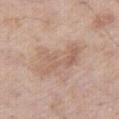Q: Lesion location?
A: the leg
Q: Illumination type?
A: white-light
Q: How was this image acquired?
A: ~15 mm crop, total-body skin-cancer survey
Q: What did automated image analysis measure?
A: a lesion area of about 12 mm² and an outline eccentricity of about 0.9 (0 = round, 1 = elongated); a detector confidence of about 100 out of 100 that the crop contains a lesion
Q: Patient demographics?
A: male, in their 60s